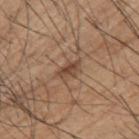body site: the left upper arm | image-analysis metrics: roughly 10 lightness units darker than nearby skin and a lesion-to-skin contrast of about 7.5 (normalized; higher = more distinct) | patient: male, aged around 55 | size: ≈2.5 mm | illumination: white-light illumination | image: 15 mm crop, total-body photography.